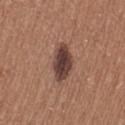Assessment:
The lesion was tiled from a total-body skin photograph and was not biopsied.
Acquisition and patient details:
This image is a 15 mm lesion crop taken from a total-body photograph. The lesion is located on the leg. Automated tile analysis of the lesion measured a border-irregularity rating of about 2/10, a within-lesion color-variation index near 4/10, and a peripheral color-asymmetry measure near 1. The software also gave a classifier nevus-likeness of about 85/100 and lesion-presence confidence of about 100/100. Imaged with white-light lighting. A female patient about 35 years old.